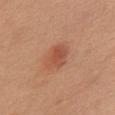The lesion was photographed on a routine skin check and not biopsied; there is no pathology result. A female subject aged approximately 70. The tile uses white-light illumination. Longest diameter approximately 3.5 mm. The lesion is located on the front of the torso. Automated image analysis of the tile measured a lesion area of about 7.5 mm², an outline eccentricity of about 0.65 (0 = round, 1 = elongated), and two-axis asymmetry of about 0.2. The software also gave a color-variation rating of about 3.5/10 and radial color variation of about 1. This image is a 15 mm lesion crop taken from a total-body photograph.